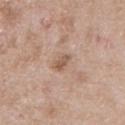biopsy status: total-body-photography surveillance lesion; no biopsy | subject: male, roughly 50 years of age | image: total-body-photography crop, ~15 mm field of view | lesion diameter: ~2.5 mm (longest diameter) | anatomic site: the chest.